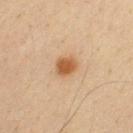– workup — no biopsy performed (imaged during a skin exam)
– tile lighting — cross-polarized
– image source — ~15 mm crop, total-body skin-cancer survey
– diameter — about 2.5 mm
– automated lesion analysis — an area of roughly 5 mm², an outline eccentricity of about 0.4 (0 = round, 1 = elongated), and a shape-asymmetry score of about 0.15 (0 = symmetric); a lesion color around L≈51 a*≈19 b*≈35 in CIELAB, about 11 CIELAB-L* units darker than the surrounding skin, and a normalized border contrast of about 9; a nevus-likeness score of about 100/100 and lesion-presence confidence of about 100/100
– location — the upper back
– subject — male, in their mid- to late 30s